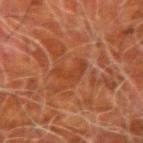Case summary:
* notes — catalogued during a skin exam; not biopsied
* imaging modality — total-body-photography crop, ~15 mm field of view
* lesion size — about 4 mm
* TBP lesion metrics — a lesion area of about 4.5 mm², an outline eccentricity of about 0.9 (0 = round, 1 = elongated), and a symmetry-axis asymmetry near 0.65; internal color variation of about 0.5 on a 0–10 scale and a peripheral color-asymmetry measure near 0; an automated nevus-likeness rating near 0 out of 100
* subject — male, aged 78 to 82
* location — the left forearm
* illumination — cross-polarized